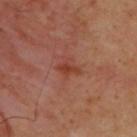workup: catalogued during a skin exam; not biopsied | image source: total-body-photography crop, ~15 mm field of view | location: the upper back | subject: male, about 45 years old | diameter: ≈2.5 mm | tile lighting: cross-polarized | TBP lesion metrics: a footprint of about 3 mm².